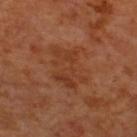notes: total-body-photography surveillance lesion; no biopsy
body site: the upper back
lighting: cross-polarized illumination
image: 15 mm crop, total-body photography
subject: male, roughly 70 years of age
size: about 5.5 mm